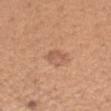Q: Was a biopsy performed?
A: catalogued during a skin exam; not biopsied
Q: Illumination type?
A: white-light
Q: What is the imaging modality?
A: total-body-photography crop, ~15 mm field of view
Q: What are the patient's age and sex?
A: female, roughly 45 years of age
Q: How large is the lesion?
A: ≈2.5 mm
Q: Where on the body is the lesion?
A: the arm